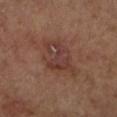No biopsy was performed on this lesion — it was imaged during a full skin examination and was not determined to be concerning. The subject is a male in their mid-60s. About 5.5 mm across. The tile uses cross-polarized illumination. A 15 mm close-up tile from a total-body photography series done for melanoma screening. Automated image analysis of the tile measured a lesion color around L≈38 a*≈20 b*≈24 in CIELAB, about 7 CIELAB-L* units darker than the surrounding skin, and a normalized border contrast of about 6. The software also gave a border-irregularity rating of about 4/10, a color-variation rating of about 5/10, and peripheral color asymmetry of about 1.5. The lesion is on the leg.